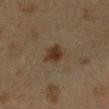  patient:
    sex: female
    age_approx: 45
  site: left forearm
  image:
    source: total-body photography crop
    field_of_view_mm: 15